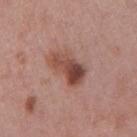| feature | finding |
|---|---|
| follow-up | no biopsy performed (imaged during a skin exam) |
| acquisition | total-body-photography crop, ~15 mm field of view |
| illumination | white-light |
| patient | female, about 40 years old |
| site | the right upper arm |